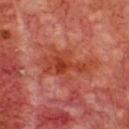  biopsy_status: not biopsied; imaged during a skin examination
  image:
    source: total-body photography crop
    field_of_view_mm: 15
  site: chest
  patient:
    sex: male
    age_approx: 70
  lighting: cross-polarized
  lesion_size:
    long_diameter_mm_approx: 6.0